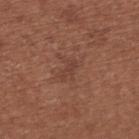  biopsy_status: not biopsied; imaged during a skin examination
  lesion_size:
    long_diameter_mm_approx: 2.5
  site: upper back
  image:
    source: total-body photography crop
    field_of_view_mm: 15
  patient:
    sex: female
    age_approx: 65